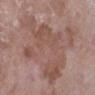Acquisition and patient details: Imaged with white-light lighting. Located on the left lower leg. A roughly 15 mm field-of-view crop from a total-body skin photograph. The lesion's longest dimension is about 11 mm. A female subject, approximately 70 years of age.